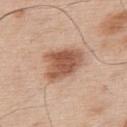| field | value |
|---|---|
| biopsy status | imaged on a skin check; not biopsied |
| size | about 5 mm |
| location | the upper back |
| patient | male, approximately 55 years of age |
| image-analysis metrics | a footprint of about 15 mm² and an eccentricity of roughly 0.6; a color-variation rating of about 4.5/10 and peripheral color asymmetry of about 1.5; a classifier nevus-likeness of about 95/100 and a lesion-detection confidence of about 100/100 |
| image | 15 mm crop, total-body photography |